From the lower back · the patient is a male approximately 60 years of age · a lesion tile, about 15 mm wide, cut from a 3D total-body photograph:
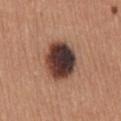This is a white-light tile. The lesion's longest dimension is about 5 mm. The lesion was biopsied, and histopathology showed a dysplastic (Clark) nevus.